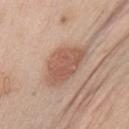follow-up — imaged on a skin check; not biopsied | lesion diameter — ~6.5 mm (longest diameter) | subject — male, aged 53–57 | lighting — white-light illumination | automated metrics — an eccentricity of roughly 0.8 and two-axis asymmetry of about 0.25 | body site — the chest | imaging modality — total-body-photography crop, ~15 mm field of view.